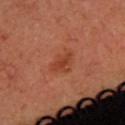Recorded during total-body skin imaging; not selected for excision or biopsy. Approximately 3.5 mm at its widest. From the upper back. A female subject, roughly 40 years of age. Automated image analysis of the tile measured a border-irregularity index near 2.5/10 and a within-lesion color-variation index near 3.5/10. And it measured a nevus-likeness score of about 25/100 and a lesion-detection confidence of about 100/100. Cropped from a whole-body photographic skin survey; the tile spans about 15 mm.